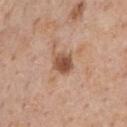• biopsy status · imaged on a skin check; not biopsied
• image source · 15 mm crop, total-body photography
• subject · male, roughly 60 years of age
• lesion diameter · about 2.5 mm
• TBP lesion metrics · a lesion area of about 5.5 mm², a shape eccentricity near 0.4, and a shape-asymmetry score of about 0.15 (0 = symmetric); an average lesion color of about L≈51 a*≈22 b*≈31 (CIELAB) and a lesion–skin lightness drop of about 13; lesion-presence confidence of about 100/100
• anatomic site · the chest
• lighting · white-light